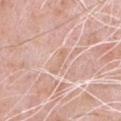Recorded during total-body skin imaging; not selected for excision or biopsy. The lesion is on the chest. A 15 mm crop from a total-body photograph taken for skin-cancer surveillance. A male subject, about 50 years old. The lesion-visualizer software estimated a lesion–skin lightness drop of about 6 and a lesion-to-skin contrast of about 4.5 (normalized; higher = more distinct). The software also gave a lesion-detection confidence of about 55/100. Longest diameter approximately 3 mm.